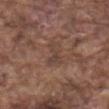Clinical impression: Part of a total-body skin-imaging series; this lesion was reviewed on a skin check and was not flagged for biopsy. Image and clinical context: A 15 mm close-up extracted from a 3D total-body photography capture. Imaged with white-light lighting. Located on the mid back. Automated tile analysis of the lesion measured a border-irregularity rating of about 5.5/10, a within-lesion color-variation index near 2/10, and radial color variation of about 0.5. A male subject approximately 75 years of age.